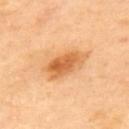follow-up: imaged on a skin check; not biopsied
body site: the upper back
acquisition: total-body-photography crop, ~15 mm field of view